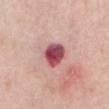Clinical impression:
No biopsy was performed on this lesion — it was imaged during a full skin examination and was not determined to be concerning.
Clinical summary:
On the abdomen. The subject is a female in their mid- to late 60s. A roughly 15 mm field-of-view crop from a total-body skin photograph.